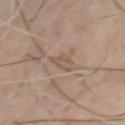follow-up: catalogued during a skin exam; not biopsied
tile lighting: cross-polarized illumination
automated lesion analysis: an average lesion color of about L≈53 a*≈14 b*≈26 (CIELAB), about 7 CIELAB-L* units darker than the surrounding skin, and a normalized border contrast of about 5.5; border irregularity of about 5 on a 0–10 scale, a color-variation rating of about 2/10, and peripheral color asymmetry of about 1
site: the chest
patient: male, approximately 65 years of age
lesion size: ~4 mm (longest diameter)
image: total-body-photography crop, ~15 mm field of view